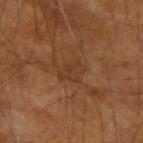| feature | finding |
|---|---|
| follow-up | no biopsy performed (imaged during a skin exam) |
| diameter | ≈3 mm |
| tile lighting | cross-polarized |
| subject | male, about 60 years old |
| acquisition | 15 mm crop, total-body photography |
| anatomic site | the arm |
| TBP lesion metrics | border irregularity of about 4 on a 0–10 scale, a within-lesion color-variation index near 2/10, and a peripheral color-asymmetry measure near 0.5 |